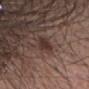Assessment: Imaged during a routine full-body skin examination; the lesion was not biopsied and no histopathology is available.